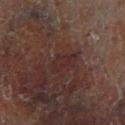Impression:
Part of a total-body skin-imaging series; this lesion was reviewed on a skin check and was not flagged for biopsy.
Acquisition and patient details:
A male patient, in their 60s. Captured under cross-polarized illumination. The recorded lesion diameter is about 3.5 mm. A 15 mm crop from a total-body photograph taken for skin-cancer surveillance. From the right lower leg.